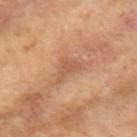TBP lesion metrics: an outline eccentricity of about 0.8 (0 = round, 1 = elongated) and two-axis asymmetry of about 0.55 | image source: ~15 mm crop, total-body skin-cancer survey | subject: female, in their mid-70s | size: about 3.5 mm | anatomic site: the right upper arm | illumination: cross-polarized illumination.